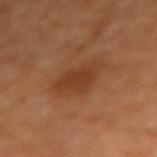TBP lesion metrics = a lesion area of about 11 mm² and a shape eccentricity near 0.75; a mean CIELAB color near L≈38 a*≈23 b*≈33, roughly 8 lightness units darker than nearby skin, and a normalized lesion–skin contrast near 6.5 | tile lighting = cross-polarized | lesion diameter = ~5 mm (longest diameter) | body site = the right forearm | subject = female, aged around 65 | image source = total-body-photography crop, ~15 mm field of view.